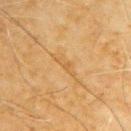Acquisition and patient details:
This image is a 15 mm lesion crop taken from a total-body photograph. The lesion is located on the left upper arm. Approximately 3 mm at its widest. The lesion-visualizer software estimated an area of roughly 3 mm², a shape eccentricity near 0.95, and a shape-asymmetry score of about 0.4 (0 = symmetric). The software also gave an average lesion color of about L≈50 a*≈16 b*≈38 (CIELAB) and a lesion–skin lightness drop of about 5. And it measured a border-irregularity index near 5/10, a color-variation rating of about 0/10, and radial color variation of about 0. A male subject, aged 68–72. Captured under cross-polarized illumination.